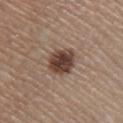The lesion was tiled from a total-body skin photograph and was not biopsied.
A 15 mm close-up tile from a total-body photography series done for melanoma screening.
Located on the right lower leg.
Longest diameter approximately 3 mm.
This is a white-light tile.
A male patient, aged around 45.